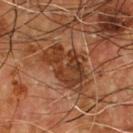{"biopsy_status": "not biopsied; imaged during a skin examination"}